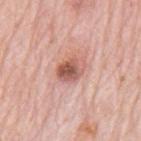workup: no biopsy performed (imaged during a skin exam) | location: the mid back | imaging modality: ~15 mm crop, total-body skin-cancer survey | TBP lesion metrics: a lesion color around L≈57 a*≈25 b*≈27 in CIELAB, a lesion–skin lightness drop of about 14, and a normalized border contrast of about 8.5; border irregularity of about 1.5 on a 0–10 scale, internal color variation of about 7.5 on a 0–10 scale, and a peripheral color-asymmetry measure near 3; an automated nevus-likeness rating near 35 out of 100 and a lesion-detection confidence of about 100/100 | illumination: white-light | subject: male, aged approximately 80 | lesion size: ~3.5 mm (longest diameter).